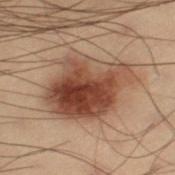Q: Was a biopsy performed?
A: catalogued during a skin exam; not biopsied
Q: Who is the patient?
A: male, aged approximately 55
Q: How large is the lesion?
A: about 8 mm
Q: Automated lesion metrics?
A: an area of roughly 33 mm², an outline eccentricity of about 0.7 (0 = round, 1 = elongated), and a shape-asymmetry score of about 0.15 (0 = symmetric)
Q: What kind of image is this?
A: ~15 mm tile from a whole-body skin photo
Q: What is the anatomic site?
A: the left thigh